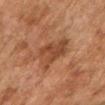Q: Is there a histopathology result?
A: no biopsy performed (imaged during a skin exam)
Q: What did automated image analysis measure?
A: a lesion area of about 11 mm² and a symmetry-axis asymmetry near 0.35; about 9 CIELAB-L* units darker than the surrounding skin; a border-irregularity index near 4/10, internal color variation of about 3 on a 0–10 scale, and radial color variation of about 1; a detector confidence of about 100 out of 100 that the crop contains a lesion
Q: What lighting was used for the tile?
A: cross-polarized
Q: Who is the patient?
A: female, aged approximately 60
Q: What is the lesion's diameter?
A: ≈4 mm
Q: Lesion location?
A: the right upper arm
Q: What kind of image is this?
A: ~15 mm tile from a whole-body skin photo